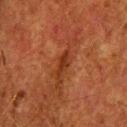image:
  source: total-body photography crop
  field_of_view_mm: 15
patient:
  sex: male
  age_approx: 65
site: head or neck
lighting: cross-polarized
lesion_size:
  long_diameter_mm_approx: 3.0